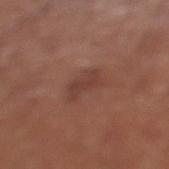Assessment:
The lesion was tiled from a total-body skin photograph and was not biopsied.
Image and clinical context:
A male subject, in their 70s. A 15 mm crop from a total-body photograph taken for skin-cancer surveillance. The lesion is on the left lower leg.